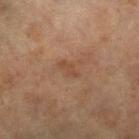Q: Is there a histopathology result?
A: catalogued during a skin exam; not biopsied
Q: What are the patient's age and sex?
A: female, roughly 65 years of age
Q: What is the anatomic site?
A: the left leg
Q: What is the imaging modality?
A: 15 mm crop, total-body photography